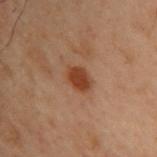Captured during whole-body skin photography for melanoma surveillance; the lesion was not biopsied.
The subject is a male roughly 55 years of age.
This is a cross-polarized tile.
The lesion is located on the back.
Cropped from a whole-body photographic skin survey; the tile spans about 15 mm.
Approximately 3 mm at its widest.